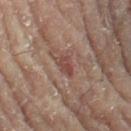No biopsy was performed on this lesion — it was imaged during a full skin examination and was not determined to be concerning.
The lesion is located on the leg.
The lesion's longest dimension is about 3 mm.
Imaged with cross-polarized lighting.
The subject is a female in their 80s.
A region of skin cropped from a whole-body photographic capture, roughly 15 mm wide.